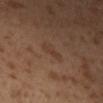Case summary:
* image — 15 mm crop, total-body photography
* location — the left leg
* subject — male, aged 28 to 32
* tile lighting — cross-polarized